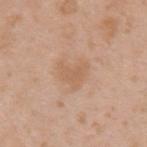Notes:
* notes: no biopsy performed (imaged during a skin exam)
* image-analysis metrics: a lesion area of about 7.5 mm² and an outline eccentricity of about 0.5 (0 = round, 1 = elongated); a lesion color around L≈61 a*≈19 b*≈33 in CIELAB, roughly 6 lightness units darker than nearby skin, and a normalized border contrast of about 5
* image source: ~15 mm tile from a whole-body skin photo
* tile lighting: white-light illumination
* patient: male, in their mid- to late 30s
* anatomic site: the left upper arm
* diameter: about 3.5 mm